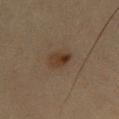Findings:
- notes: total-body-photography surveillance lesion; no biopsy
- patient: male, aged 33 to 37
- body site: the front of the torso
- tile lighting: cross-polarized illumination
- acquisition: 15 mm crop, total-body photography